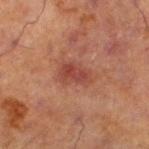biopsy_status: not biopsied; imaged during a skin examination
lesion_size:
  long_diameter_mm_approx: 3.5
site: left lower leg
image:
  source: total-body photography crop
  field_of_view_mm: 15
patient:
  sex: male
  age_approx: 70
lighting: cross-polarized
automated_metrics:
  eccentricity: 0.8
  shape_asymmetry: 0.3
  border_irregularity_0_10: 3.0
  color_variation_0_10: 3.0